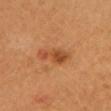notes: catalogued during a skin exam; not biopsied
lesion size: ≈4.5 mm
site: the chest
image: 15 mm crop, total-body photography
lighting: cross-polarized illumination
patient: female, approximately 55 years of age